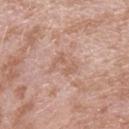Impression: This lesion was catalogued during total-body skin photography and was not selected for biopsy. Acquisition and patient details: From the left forearm. This is a white-light tile. A lesion tile, about 15 mm wide, cut from a 3D total-body photograph. The lesion-visualizer software estimated a footprint of about 4.5 mm², an eccentricity of roughly 0.8, and two-axis asymmetry of about 0.45. It also reported an average lesion color of about L≈60 a*≈20 b*≈28 (CIELAB), roughly 7 lightness units darker than nearby skin, and a normalized border contrast of about 5. The analysis additionally found a border-irregularity index near 8/10, internal color variation of about 2 on a 0–10 scale, and peripheral color asymmetry of about 1. It also reported a nevus-likeness score of about 0/100. A male subject about 40 years old.